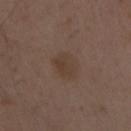• workup · imaged on a skin check; not biopsied
• image source · ~15 mm tile from a whole-body skin photo
• anatomic site · the left forearm
• TBP lesion metrics · an outline eccentricity of about 0.55 (0 = round, 1 = elongated); border irregularity of about 1.5 on a 0–10 scale and a peripheral color-asymmetry measure near 1; a nevus-likeness score of about 10/100 and lesion-presence confidence of about 100/100
• subject · male, aged 48–52